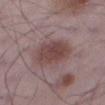follow-up: catalogued during a skin exam; not biopsied
lighting: white-light
body site: the right thigh
patient: male, aged 48–52
image: total-body-photography crop, ~15 mm field of view
diameter: ~6 mm (longest diameter)